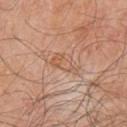Case summary:
- notes — catalogued during a skin exam; not biopsied
- image source — ~15 mm crop, total-body skin-cancer survey
- subject — male, about 80 years old
- anatomic site — the right upper arm
- automated metrics — an area of roughly 4.5 mm², an eccentricity of roughly 0.9, and a symmetry-axis asymmetry near 0.45; about 6 CIELAB-L* units darker than the surrounding skin and a normalized border contrast of about 5; a nevus-likeness score of about 0/100
- size — ~3.5 mm (longest diameter)